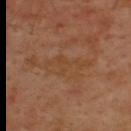Image and clinical context: Automated image analysis of the tile measured a lesion area of about 8 mm² and a shape-asymmetry score of about 0.5 (0 = symmetric). The software also gave a lesion–skin lightness drop of about 4 and a normalized lesion–skin contrast near 4.5. The analysis additionally found internal color variation of about 2.5 on a 0–10 scale and radial color variation of about 0.5. A close-up tile cropped from a whole-body skin photograph, about 15 mm across. Located on the back. Approximately 6 mm at its widest. A male subject, aged around 45.